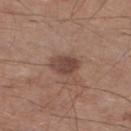Notes:
– biopsy status · catalogued during a skin exam; not biopsied
– diameter · about 3.5 mm
– image · ~15 mm crop, total-body skin-cancer survey
– illumination · white-light illumination
– body site · the right lower leg
– patient · male, in their 60s